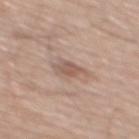{
  "biopsy_status": "not biopsied; imaged during a skin examination",
  "patient": {
    "sex": "male",
    "age_approx": 65
  },
  "lighting": "white-light",
  "site": "mid back",
  "lesion_size": {
    "long_diameter_mm_approx": 3.5
  },
  "image": {
    "source": "total-body photography crop",
    "field_of_view_mm": 15
  }
}